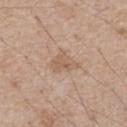The lesion was photographed on a routine skin check and not biopsied; there is no pathology result. The recorded lesion diameter is about 3 mm. An algorithmic analysis of the crop reported a footprint of about 5.5 mm², an outline eccentricity of about 0.65 (0 = round, 1 = elongated), and a symmetry-axis asymmetry near 0.35. It also reported a border-irregularity index near 3.5/10, internal color variation of about 2.5 on a 0–10 scale, and peripheral color asymmetry of about 1. The software also gave a nevus-likeness score of about 0/100. Captured under white-light illumination. On the chest. The patient is a male approximately 65 years of age. Cropped from a whole-body photographic skin survey; the tile spans about 15 mm.